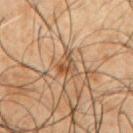workup: imaged on a skin check; not biopsied | body site: the arm | image source: ~15 mm crop, total-body skin-cancer survey | lesion diameter: about 2.5 mm | patient: male, approximately 50 years of age | tile lighting: cross-polarized illumination.